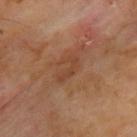Q: Is there a histopathology result?
A: no biopsy performed (imaged during a skin exam)
Q: Where on the body is the lesion?
A: the upper back
Q: What are the patient's age and sex?
A: male, aged approximately 70
Q: How was this image acquired?
A: total-body-photography crop, ~15 mm field of view